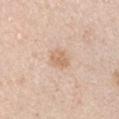Q: What did automated image analysis measure?
A: a mean CIELAB color near L≈67 a*≈17 b*≈32 and about 8 CIELAB-L* units darker than the surrounding skin; border irregularity of about 1 on a 0–10 scale, a within-lesion color-variation index near 2/10, and peripheral color asymmetry of about 0.5
Q: Lesion location?
A: the left upper arm
Q: How was this image acquired?
A: ~15 mm tile from a whole-body skin photo
Q: Patient demographics?
A: male, about 40 years old
Q: Lesion size?
A: ≈2.5 mm